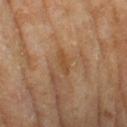Impression:
No biopsy was performed on this lesion — it was imaged during a full skin examination and was not determined to be concerning.
Background:
A female subject about 60 years old. Cropped from a total-body skin-imaging series; the visible field is about 15 mm. The lesion is on the leg.